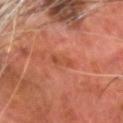The lesion was tiled from a total-body skin photograph and was not biopsied.
A male patient aged approximately 70.
A lesion tile, about 15 mm wide, cut from a 3D total-body photograph.
On the head or neck.